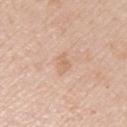Approximately 3 mm at its widest. A 15 mm close-up tile from a total-body photography series done for melanoma screening. A female patient, aged around 45. Captured under white-light illumination. The lesion is on the left upper arm.